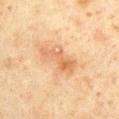biopsy_status: not biopsied; imaged during a skin examination
lesion_size:
  long_diameter_mm_approx: 5.0
patient:
  sex: male
  age_approx: 60
site: front of the torso
lighting: cross-polarized
image:
  source: total-body photography crop
  field_of_view_mm: 15